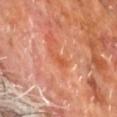workup: total-body-photography surveillance lesion; no biopsy
patient: male, approximately 60 years of age
lesion size: ~3 mm (longest diameter)
tile lighting: cross-polarized illumination
anatomic site: the back
image source: ~15 mm tile from a whole-body skin photo